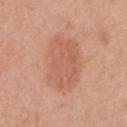Part of a total-body skin-imaging series; this lesion was reviewed on a skin check and was not flagged for biopsy.
From the right upper arm.
The tile uses white-light illumination.
This image is a 15 mm lesion crop taken from a total-body photograph.
A female patient about 55 years old.
Automated tile analysis of the lesion measured border irregularity of about 2 on a 0–10 scale, internal color variation of about 3 on a 0–10 scale, and a peripheral color-asymmetry measure near 1. It also reported a classifier nevus-likeness of about 10/100 and a detector confidence of about 100 out of 100 that the crop contains a lesion.
Measured at roughly 7 mm in maximum diameter.